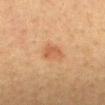Captured during whole-body skin photography for melanoma surveillance; the lesion was not biopsied. From the mid back. A region of skin cropped from a whole-body photographic capture, roughly 15 mm wide. Approximately 3 mm at its widest. A male patient, aged around 35. Captured under cross-polarized illumination.